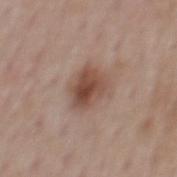Impression:
Recorded during total-body skin imaging; not selected for excision or biopsy.
Acquisition and patient details:
Captured under white-light illumination. Longest diameter approximately 4 mm. Cropped from a whole-body photographic skin survey; the tile spans about 15 mm. A male patient, aged 53 to 57. The lesion is located on the mid back. The total-body-photography lesion software estimated a lesion area of about 10 mm², a shape eccentricity near 0.65, and a symmetry-axis asymmetry near 0.25.